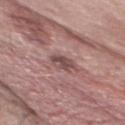Findings:
– image-analysis metrics — a lesion area of about 5 mm², an outline eccentricity of about 0.7 (0 = round, 1 = elongated), and a symmetry-axis asymmetry near 0.25; an average lesion color of about L≈48 a*≈20 b*≈19 (CIELAB) and a normalized border contrast of about 8; a nevus-likeness score of about 15/100 and a lesion-detection confidence of about 100/100
– subject — male, in their mid- to late 50s
– lighting — white-light illumination
– imaging modality — ~15 mm tile from a whole-body skin photo
– location — the chest
– diameter — ~3 mm (longest diameter)